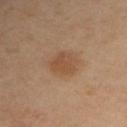Case summary:
- follow-up · no biopsy performed (imaged during a skin exam)
- diameter · ≈3.5 mm
- lighting · cross-polarized illumination
- site · the right upper arm
- acquisition · ~15 mm tile from a whole-body skin photo
- patient · male, approximately 40 years of age